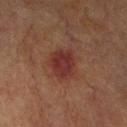follow-up: imaged on a skin check; not biopsied
lighting: cross-polarized illumination
image: ~15 mm crop, total-body skin-cancer survey
subject: male, approximately 75 years of age
lesion size: ≈3.5 mm
body site: the right upper arm